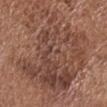Captured during whole-body skin photography for melanoma surveillance; the lesion was not biopsied. A male subject roughly 75 years of age. This image is a 15 mm lesion crop taken from a total-body photograph. The recorded lesion diameter is about 15.5 mm. From the head or neck. Imaged with white-light lighting.